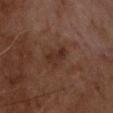Imaged during a routine full-body skin examination; the lesion was not biopsied and no histopathology is available.